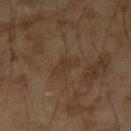Clinical impression: Recorded during total-body skin imaging; not selected for excision or biopsy. Acquisition and patient details: The patient is a male approximately 45 years of age. The recorded lesion diameter is about 3 mm. A 15 mm close-up extracted from a 3D total-body photography capture. Located on the left forearm. The tile uses cross-polarized illumination.